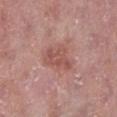notes = catalogued during a skin exam; not biopsied | automated lesion analysis = an automated nevus-likeness rating near 0 out of 100 and a detector confidence of about 100 out of 100 that the crop contains a lesion | anatomic site = the left lower leg | lesion size = ~4.5 mm (longest diameter) | tile lighting = white-light | subject = male, roughly 55 years of age | image = total-body-photography crop, ~15 mm field of view.